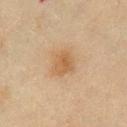Imaged during a routine full-body skin examination; the lesion was not biopsied and no histopathology is available. A male patient, aged approximately 70. A close-up tile cropped from a whole-body skin photograph, about 15 mm across. The lesion is located on the chest. The recorded lesion diameter is about 3 mm. The tile uses cross-polarized illumination.